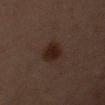Background:
Approximately 3.5 mm at its widest. A roughly 15 mm field-of-view crop from a total-body skin photograph. Located on the right upper arm. Imaged with cross-polarized lighting. The subject is a male roughly 60 years of age. The lesion-visualizer software estimated an area of roughly 7.5 mm², an outline eccentricity of about 0.75 (0 = round, 1 = elongated), and two-axis asymmetry of about 0.15. The software also gave lesion-presence confidence of about 100/100.